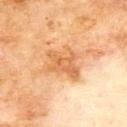A lesion tile, about 15 mm wide, cut from a 3D total-body photograph.
The patient is a male aged 68–72.
Longest diameter approximately 4.5 mm.
The lesion is on the upper back.